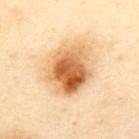| field | value |
|---|---|
| follow-up | no biopsy performed (imaged during a skin exam) |
| acquisition | ~15 mm tile from a whole-body skin photo |
| body site | the upper back |
| lighting | cross-polarized |
| patient | female, in their 40s |
| diameter | about 6.5 mm |